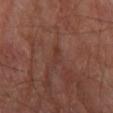workup: total-body-photography surveillance lesion; no biopsy
lighting: cross-polarized
image: total-body-photography crop, ~15 mm field of view
body site: the left lower leg
subject: male, aged 68 to 72
automated metrics: an average lesion color of about L≈34 a*≈22 b*≈25 (CIELAB), about 5 CIELAB-L* units darker than the surrounding skin, and a normalized lesion–skin contrast near 5; a nevus-likeness score of about 0/100 and a lesion-detection confidence of about 55/100
lesion size: ~2.5 mm (longest diameter)